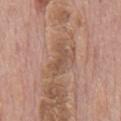| feature | finding |
|---|---|
| patient | male, roughly 75 years of age |
| location | the back |
| automated metrics | a footprint of about 5.5 mm², an eccentricity of roughly 0.9, and a symmetry-axis asymmetry near 0.4; a mean CIELAB color near L≈52 a*≈18 b*≈28, about 8 CIELAB-L* units darker than the surrounding skin, and a normalized border contrast of about 6; a nevus-likeness score of about 0/100 and a lesion-detection confidence of about 90/100 |
| lesion diameter | about 4 mm |
| acquisition | 15 mm crop, total-body photography |